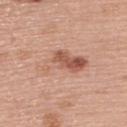Cropped from a whole-body photographic skin survey; the tile spans about 15 mm.
The subject is a female in their 60s.
The lesion is on the upper back.
Longest diameter approximately 6.5 mm.